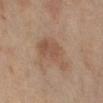notes: imaged on a skin check; not biopsied | location: the right lower leg | automated lesion analysis: a footprint of about 11 mm² and two-axis asymmetry of about 0.45; a mean CIELAB color near L≈43 a*≈16 b*≈25 and a lesion-to-skin contrast of about 6 (normalized; higher = more distinct); a border-irregularity index near 5/10, a within-lesion color-variation index near 2.5/10, and peripheral color asymmetry of about 0.5; a nevus-likeness score of about 5/100 and a lesion-detection confidence of about 100/100 | diameter: ≈5.5 mm | subject: female, aged 68 to 72 | image: 15 mm crop, total-body photography.